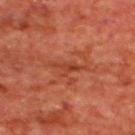The lesion was photographed on a routine skin check and not biopsied; there is no pathology result.
Located on the upper back.
A male patient, about 65 years old.
This image is a 15 mm lesion crop taken from a total-body photograph.